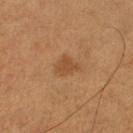Impression: This lesion was catalogued during total-body skin photography and was not selected for biopsy. Acquisition and patient details: The lesion's longest dimension is about 3 mm. This image is a 15 mm lesion crop taken from a total-body photograph. The total-body-photography lesion software estimated a lesion area of about 4.5 mm², a shape eccentricity near 0.65, and a symmetry-axis asymmetry near 0.35. It also reported a lesion–skin lightness drop of about 7 and a normalized lesion–skin contrast near 6. The analysis additionally found an automated nevus-likeness rating near 40 out of 100 and a detector confidence of about 100 out of 100 that the crop contains a lesion. The lesion is located on the left lower leg. A male subject, aged 53–57. This is a cross-polarized tile.